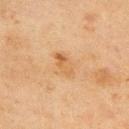notes: imaged on a skin check; not biopsied | image source: 15 mm crop, total-body photography | TBP lesion metrics: an average lesion color of about L≈50 a*≈18 b*≈35 (CIELAB) and roughly 7 lightness units darker than nearby skin; border irregularity of about 2 on a 0–10 scale and peripheral color asymmetry of about 2.5 | site: the upper back | tile lighting: cross-polarized illumination | patient: male, in their mid- to late 40s.